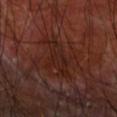Q: What is the lesion's diameter?
A: ≈5.5 mm
Q: How was the tile lit?
A: cross-polarized
Q: Lesion location?
A: the right upper arm
Q: How was this image acquired?
A: total-body-photography crop, ~15 mm field of view
Q: What are the patient's age and sex?
A: male, aged 58 to 62